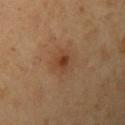Assessment:
Captured during whole-body skin photography for melanoma surveillance; the lesion was not biopsied.
Clinical summary:
Automated image analysis of the tile measured a footprint of about 3 mm² and two-axis asymmetry of about 0.2. The analysis additionally found a nevus-likeness score of about 30/100. Located on the arm. The tile uses cross-polarized illumination. A female subject, about 45 years old. About 2 mm across. A 15 mm close-up tile from a total-body photography series done for melanoma screening.